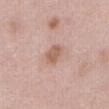Captured during whole-body skin photography for melanoma surveillance; the lesion was not biopsied. The tile uses white-light illumination. About 2.5 mm across. The lesion-visualizer software estimated a nevus-likeness score of about 15/100 and a detector confidence of about 100 out of 100 that the crop contains a lesion. The lesion is located on the front of the torso. A female subject, aged around 40. A 15 mm crop from a total-body photograph taken for skin-cancer surveillance.